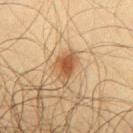| field | value |
|---|---|
| biopsy status | catalogued during a skin exam; not biopsied |